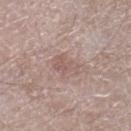Clinical impression: Part of a total-body skin-imaging series; this lesion was reviewed on a skin check and was not flagged for biopsy. Clinical summary: Cropped from a total-body skin-imaging series; the visible field is about 15 mm. A male patient aged approximately 65. The lesion is on the right lower leg.